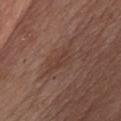<record>
  <biopsy_status>not biopsied; imaged during a skin examination</biopsy_status>
  <site>chest</site>
  <patient>
    <sex>male</sex>
    <age_approx>55</age_approx>
  </patient>
  <image>
    <source>total-body photography crop</source>
    <field_of_view_mm>15</field_of_view_mm>
  </image>
  <lesion_size>
    <long_diameter_mm_approx>4.5</long_diameter_mm_approx>
  </lesion_size>
</record>